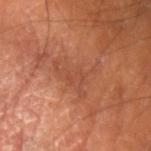• biopsy status: total-body-photography surveillance lesion; no biopsy
• anatomic site: the left upper arm
• automated metrics: a lesion area of about 8.5 mm², an outline eccentricity of about 0.8 (0 = round, 1 = elongated), and a shape-asymmetry score of about 0.65 (0 = symmetric); a peripheral color-asymmetry measure near 0.5
• acquisition: 15 mm crop, total-body photography
• subject: male, approximately 60 years of age
• size: ~5 mm (longest diameter)
• illumination: cross-polarized